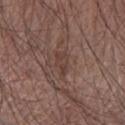Measured at roughly 2.5 mm in maximum diameter. The lesion is located on the right forearm. A 15 mm close-up extracted from a 3D total-body photography capture. This is a white-light tile. The subject is a male in their mid-60s. Automated image analysis of the tile measured an area of roughly 3.5 mm² and two-axis asymmetry of about 0.4. The software also gave an average lesion color of about L≈39 a*≈17 b*≈22 (CIELAB) and a normalized border contrast of about 5.5.